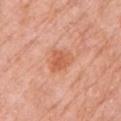Notes:
– biopsy status · imaged on a skin check; not biopsied
– patient · female, roughly 75 years of age
– body site · the left upper arm
– lesion size · about 3 mm
– imaging modality · ~15 mm tile from a whole-body skin photo